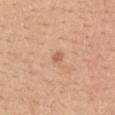Q: Is there a histopathology result?
A: catalogued during a skin exam; not biopsied
Q: How was this image acquired?
A: ~15 mm tile from a whole-body skin photo
Q: Lesion size?
A: ≈1.5 mm
Q: What did automated image analysis measure?
A: a lesion area of about 1.5 mm² and an eccentricity of roughly 0.65; a color-variation rating of about 0/10 and radial color variation of about 0; a classifier nevus-likeness of about 0/100
Q: What lighting was used for the tile?
A: white-light
Q: Patient demographics?
A: female, in their mid-40s
Q: Lesion location?
A: the upper back